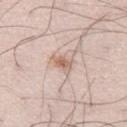Part of a total-body skin-imaging series; this lesion was reviewed on a skin check and was not flagged for biopsy.
A lesion tile, about 15 mm wide, cut from a 3D total-body photograph.
Imaged with white-light lighting.
The lesion is located on the left thigh.
A male patient, about 35 years old.
The lesion's longest dimension is about 2.5 mm.